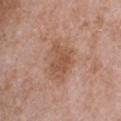This lesion was catalogued during total-body skin photography and was not selected for biopsy. From the front of the torso. The tile uses white-light illumination. A lesion tile, about 15 mm wide, cut from a 3D total-body photograph. A male subject aged 48–52.